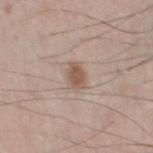lesion diameter=about 3 mm | acquisition=~15 mm tile from a whole-body skin photo | illumination=white-light illumination | subject=male, roughly 60 years of age | location=the left thigh.